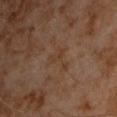Imaged during a routine full-body skin examination; the lesion was not biopsied and no histopathology is available.
Cropped from a total-body skin-imaging series; the visible field is about 15 mm.
Captured under cross-polarized illumination.
A male patient, aged around 60.
The lesion's longest dimension is about 3 mm.
Located on the chest.